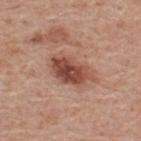Part of a total-body skin-imaging series; this lesion was reviewed on a skin check and was not flagged for biopsy. The subject is a male roughly 60 years of age. The lesion is on the upper back. Measured at roughly 5.5 mm in maximum diameter. This is a white-light tile. A region of skin cropped from a whole-body photographic capture, roughly 15 mm wide.